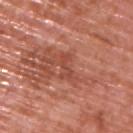Imaged during a routine full-body skin examination; the lesion was not biopsied and no histopathology is available. A 15 mm close-up tile from a total-body photography series done for melanoma screening. The patient is a male aged 68–72. The lesion is located on the upper back. Imaged with white-light lighting. The lesion-visualizer software estimated a mean CIELAB color near L≈48 a*≈29 b*≈31, roughly 7 lightness units darker than nearby skin, and a normalized border contrast of about 5.5. The lesion's longest dimension is about 3 mm.